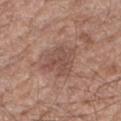Q: Was this lesion biopsied?
A: total-body-photography surveillance lesion; no biopsy
Q: What did automated image analysis measure?
A: a border-irregularity rating of about 4/10, internal color variation of about 2.5 on a 0–10 scale, and a peripheral color-asymmetry measure near 1
Q: Lesion size?
A: about 4 mm
Q: Illumination type?
A: white-light illumination
Q: Where on the body is the lesion?
A: the right forearm
Q: Patient demographics?
A: male, in their 60s
Q: How was this image acquired?
A: total-body-photography crop, ~15 mm field of view